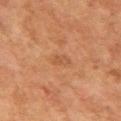| field | value |
|---|---|
| site | the arm |
| diameter | about 2.5 mm |
| lighting | cross-polarized illumination |
| acquisition | 15 mm crop, total-body photography |
| subject | female, roughly 60 years of age |
| TBP lesion metrics | an eccentricity of roughly 0.9 and a symmetry-axis asymmetry near 0.2; border irregularity of about 2 on a 0–10 scale, internal color variation of about 2 on a 0–10 scale, and peripheral color asymmetry of about 0.5; a nevus-likeness score of about 0/100 and a detector confidence of about 100 out of 100 that the crop contains a lesion |